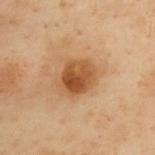<case>
  <biopsy_status>not biopsied; imaged during a skin examination</biopsy_status>
  <lesion_size>
    <long_diameter_mm_approx>4.0</long_diameter_mm_approx>
  </lesion_size>
  <lighting>cross-polarized</lighting>
  <patient>
    <sex>female</sex>
    <age_approx>60</age_approx>
  </patient>
  <image>
    <source>total-body photography crop</source>
    <field_of_view_mm>15</field_of_view_mm>
  </image>
  <site>upper back</site>
  <automated_metrics>
    <area_mm2_approx>11.0</area_mm2_approx>
    <eccentricity>0.55</eccentricity>
    <shape_asymmetry>0.15</shape_asymmetry>
    <border_irregularity_0_10>1.5</border_irregularity_0_10>
    <color_variation_0_10>6.5</color_variation_0_10>
    <peripheral_color_asymmetry>2.5</peripheral_color_asymmetry>
    <nevus_likeness_0_100>95</nevus_likeness_0_100>
    <lesion_detection_confidence_0_100>100</lesion_detection_confidence_0_100>
  </automated_metrics>
</case>